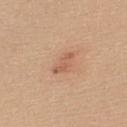Findings:
• workup — imaged on a skin check; not biopsied
• location — the back
• lighting — white-light illumination
• imaging modality — total-body-photography crop, ~15 mm field of view
• subject — female, aged 18 to 22